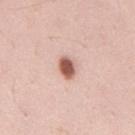Q: Was this lesion biopsied?
A: no biopsy performed (imaged during a skin exam)
Q: What are the patient's age and sex?
A: male, aged 33–37
Q: Where on the body is the lesion?
A: the back
Q: What is the imaging modality?
A: total-body-photography crop, ~15 mm field of view
Q: Automated lesion metrics?
A: a lesion area of about 4.5 mm² and a symmetry-axis asymmetry near 0.15; a nevus-likeness score of about 100/100